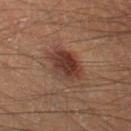Clinical summary: The patient is a male aged approximately 40. Located on the leg. This image is a 15 mm lesion crop taken from a total-body photograph. Approximately 5 mm at its widest. This is a cross-polarized tile. Automated tile analysis of the lesion measured about 10 CIELAB-L* units darker than the surrounding skin and a normalized border contrast of about 9.5.